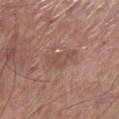biopsy status — catalogued during a skin exam; not biopsied | image — ~15 mm tile from a whole-body skin photo | lesion size — about 3.5 mm | subject — male, in their 70s | tile lighting — white-light illumination | site — the leg.